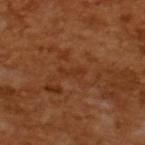Measured at roughly 3 mm in maximum diameter.
A region of skin cropped from a whole-body photographic capture, roughly 15 mm wide.
A male patient, in their mid-60s.
Captured under cross-polarized illumination.